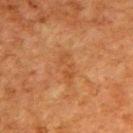Recorded during total-body skin imaging; not selected for excision or biopsy. Approximately 3.5 mm at its widest. A male subject roughly 80 years of age. From the upper back. A roughly 15 mm field-of-view crop from a total-body skin photograph.